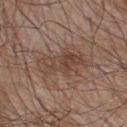workup: catalogued during a skin exam; not biopsied | image source: 15 mm crop, total-body photography | diameter: about 7 mm | site: the upper back | lighting: white-light | patient: male, aged 53–57 | TBP lesion metrics: a shape-asymmetry score of about 0.35 (0 = symmetric); an average lesion color of about L≈45 a*≈17 b*≈25 (CIELAB), about 8 CIELAB-L* units darker than the surrounding skin, and a lesion-to-skin contrast of about 6 (normalized; higher = more distinct); a color-variation rating of about 4.5/10 and radial color variation of about 1.5.